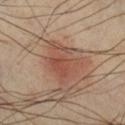Captured during whole-body skin photography for melanoma surveillance; the lesion was not biopsied. Automated image analysis of the tile measured an area of roughly 13 mm², an eccentricity of roughly 0.7, and a shape-asymmetry score of about 0.4 (0 = symmetric). And it measured a color-variation rating of about 4.5/10 and a peripheral color-asymmetry measure near 1.5. It also reported an automated nevus-likeness rating near 80 out of 100 and a lesion-detection confidence of about 100/100. This is a cross-polarized tile. This image is a 15 mm lesion crop taken from a total-body photograph. A male patient about 40 years old. On the right lower leg. The recorded lesion diameter is about 5.5 mm.